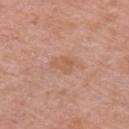No biopsy was performed on this lesion — it was imaged during a full skin examination and was not determined to be concerning.
Measured at roughly 2.5 mm in maximum diameter.
Captured under white-light illumination.
The total-body-photography lesion software estimated a footprint of about 4 mm², a shape eccentricity near 0.7, and a shape-asymmetry score of about 0.35 (0 = symmetric). And it measured a border-irregularity index near 3.5/10, internal color variation of about 2 on a 0–10 scale, and peripheral color asymmetry of about 0.5.
A male patient aged 68 to 72.
On the arm.
A 15 mm close-up tile from a total-body photography series done for melanoma screening.